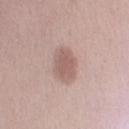biopsy_status: not biopsied; imaged during a skin examination
patient:
  sex: male
  age_approx: 60
lesion_size:
  long_diameter_mm_approx: 4.0
image:
  source: total-body photography crop
  field_of_view_mm: 15
automated_metrics:
  vs_skin_contrast_norm: 6.5
  border_irregularity_0_10: 1.5
  color_variation_0_10: 2.0
  peripheral_color_asymmetry: 0.5
  nevus_likeness_0_100: 30
site: right upper arm